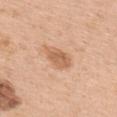follow-up: imaged on a skin check; not biopsied | image source: ~15 mm crop, total-body skin-cancer survey | TBP lesion metrics: a normalized border contrast of about 7; an automated nevus-likeness rating near 25 out of 100 and a detector confidence of about 100 out of 100 that the crop contains a lesion | subject: female, aged 63 to 67 | lighting: white-light | size: about 4 mm | site: the upper back.